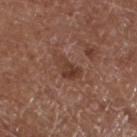Assessment:
This lesion was catalogued during total-body skin photography and was not selected for biopsy.
Clinical summary:
A male patient, aged approximately 75. Measured at roughly 3.5 mm in maximum diameter. Imaged with white-light lighting. A close-up tile cropped from a whole-body skin photograph, about 15 mm across. The total-body-photography lesion software estimated an average lesion color of about L≈38 a*≈21 b*≈27 (CIELAB) and a lesion-to-skin contrast of about 7 (normalized; higher = more distinct). It also reported a nevus-likeness score of about 5/100 and lesion-presence confidence of about 100/100. The lesion is on the left lower leg.